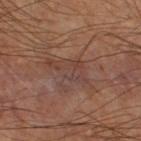Clinical impression:
No biopsy was performed on this lesion — it was imaged during a full skin examination and was not determined to be concerning.
Clinical summary:
The subject is a male in their 60s. Imaged with cross-polarized lighting. A 15 mm close-up extracted from a 3D total-body photography capture. Located on the right thigh. The recorded lesion diameter is about 6 mm.